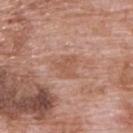This lesion was catalogued during total-body skin photography and was not selected for biopsy.